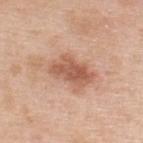Q: Was a biopsy performed?
A: no biopsy performed (imaged during a skin exam)
Q: Patient demographics?
A: male, in their 50s
Q: Illumination type?
A: white-light illumination
Q: What is the anatomic site?
A: the upper back
Q: What is the imaging modality?
A: ~15 mm crop, total-body skin-cancer survey